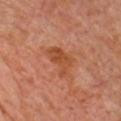  biopsy_status: not biopsied; imaged during a skin examination
  lesion_size:
    long_diameter_mm_approx: 4.5
  patient:
    sex: female
    age_approx: 60
  site: chest
  image:
    source: total-body photography crop
    field_of_view_mm: 15
  automated_metrics:
    area_mm2_approx: 7.5
    eccentricity: 0.85
    shape_asymmetry: 0.45